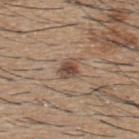Q: Was a biopsy performed?
A: no biopsy performed (imaged during a skin exam)
Q: Who is the patient?
A: male, roughly 60 years of age
Q: How large is the lesion?
A: ≈3 mm
Q: Lesion location?
A: the upper back
Q: What kind of image is this?
A: 15 mm crop, total-body photography